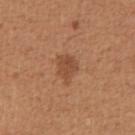Recorded during total-body skin imaging; not selected for excision or biopsy. The lesion is on the abdomen. A male subject about 55 years old. A region of skin cropped from a whole-body photographic capture, roughly 15 mm wide.